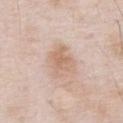patient: male, in their mid- to late 50s
automated lesion analysis: a lesion area of about 9 mm², an outline eccentricity of about 0.7 (0 = round, 1 = elongated), and a symmetry-axis asymmetry near 0.3; a nevus-likeness score of about 5/100 and a detector confidence of about 100 out of 100 that the crop contains a lesion
site: the chest
lighting: white-light
image: total-body-photography crop, ~15 mm field of view